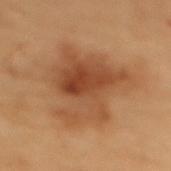  biopsy_status: not biopsied; imaged during a skin examination
  patient:
    sex: female
    age_approx: 50
  site: upper back
  image:
    source: total-body photography crop
    field_of_view_mm: 15
  lighting: cross-polarized
  lesion_size:
    long_diameter_mm_approx: 9.0